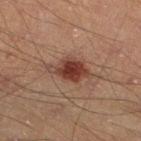The lesion was photographed on a routine skin check and not biopsied; there is no pathology result. Automated image analysis of the tile measured a classifier nevus-likeness of about 100/100 and a lesion-detection confidence of about 100/100. A 15 mm close-up extracted from a 3D total-body photography capture. Approximately 5 mm at its widest. From the leg. A male patient aged 38 to 42. Captured under cross-polarized illumination.